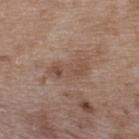Imaged with white-light lighting.
Longest diameter approximately 4.5 mm.
A lesion tile, about 15 mm wide, cut from a 3D total-body photograph.
A male subject roughly 50 years of age.
The lesion is located on the back.
The lesion-visualizer software estimated a classifier nevus-likeness of about 0/100.